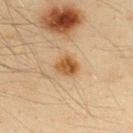Clinical impression:
The lesion was photographed on a routine skin check and not biopsied; there is no pathology result.
Clinical summary:
The tile uses cross-polarized illumination. A male subject aged 33–37. Cropped from a whole-body photographic skin survey; the tile spans about 15 mm. The recorded lesion diameter is about 2.5 mm. The lesion is located on the back. Automated tile analysis of the lesion measured a lesion area of about 5.5 mm², an eccentricity of roughly 0.55, and a symmetry-axis asymmetry near 0.15. It also reported a border-irregularity rating of about 1.5/10 and peripheral color asymmetry of about 1.5.